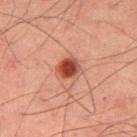biopsy_status: not biopsied; imaged during a skin examination
patient:
  sex: male
  age_approx: 60
lesion_size:
  long_diameter_mm_approx: 2.5
automated_metrics:
  border_irregularity_0_10: 1.5
  color_variation_0_10: 4.0
  peripheral_color_asymmetry: 1.0
  nevus_likeness_0_100: 100
  lesion_detection_confidence_0_100: 100
lighting: cross-polarized
image:
  source: total-body photography crop
  field_of_view_mm: 15
site: right thigh